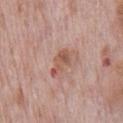<lesion>
<biopsy_status>not biopsied; imaged during a skin examination</biopsy_status>
<image>
  <source>total-body photography crop</source>
  <field_of_view_mm>15</field_of_view_mm>
</image>
<patient>
  <sex>male</sex>
  <age_approx>70</age_approx>
</patient>
<site>chest</site>
</lesion>